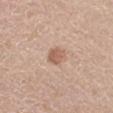workup = imaged on a skin check; not biopsied | TBP lesion metrics = a footprint of about 4 mm²; about 10 CIELAB-L* units darker than the surrounding skin and a normalized lesion–skin contrast near 7; a border-irregularity rating of about 2/10, a color-variation rating of about 2/10, and a peripheral color-asymmetry measure near 1; an automated nevus-likeness rating near 70 out of 100 and a detector confidence of about 100 out of 100 that the crop contains a lesion | location = the right lower leg | illumination = white-light illumination | subject = female, approximately 70 years of age | imaging modality = ~15 mm crop, total-body skin-cancer survey.